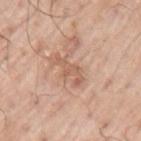<tbp_lesion>
  <biopsy_status>not biopsied; imaged during a skin examination</biopsy_status>
  <patient>
    <sex>male</sex>
    <age_approx>70</age_approx>
  </patient>
  <image>
    <source>total-body photography crop</source>
    <field_of_view_mm>15</field_of_view_mm>
  </image>
  <lighting>white-light</lighting>
  <site>left upper arm</site>
  <lesion_size>
    <long_diameter_mm_approx>4.0</long_diameter_mm_approx>
  </lesion_size>
  <automated_metrics>
    <area_mm2_approx>5.5</area_mm2_approx>
    <eccentricity>0.9</eccentricity>
    <cielab_L>60</cielab_L>
    <cielab_a>21</cielab_a>
    <cielab_b>30</cielab_b>
    <vs_skin_darker_L>8.0</vs_skin_darker_L>
    <border_irregularity_0_10>6.5</border_irregularity_0_10>
    <peripheral_color_asymmetry>0.5</peripheral_color_asymmetry>
  </automated_metrics>
</tbp_lesion>